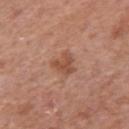workup: no biopsy performed (imaged during a skin exam)
illumination: white-light
image source: 15 mm crop, total-body photography
image-analysis metrics: an area of roughly 5 mm² and a shape eccentricity near 0.55; a lesion color around L≈50 a*≈23 b*≈31 in CIELAB, a lesion–skin lightness drop of about 9, and a normalized lesion–skin contrast near 7; internal color variation of about 2 on a 0–10 scale and peripheral color asymmetry of about 1; a classifier nevus-likeness of about 35/100 and a lesion-detection confidence of about 100/100
patient: male, aged around 65
location: the arm
lesion size: about 2.5 mm